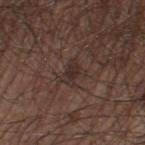  biopsy_status: not biopsied; imaged during a skin examination
  site: left thigh
  patient:
    sex: male
    age_approx: 60
  image:
    source: total-body photography crop
    field_of_view_mm: 15